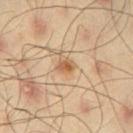Clinical impression: No biopsy was performed on this lesion — it was imaged during a full skin examination and was not determined to be concerning. Acquisition and patient details: Automated image analysis of the tile measured a lesion area of about 3 mm² and an outline eccentricity of about 0.65 (0 = round, 1 = elongated). The analysis additionally found roughly 11 lightness units darker than nearby skin and a lesion-to-skin contrast of about 7.5 (normalized; higher = more distinct). And it measured border irregularity of about 1.5 on a 0–10 scale. Located on the leg. The subject is a male approximately 45 years of age. This image is a 15 mm lesion crop taken from a total-body photograph. About 2 mm across. Imaged with cross-polarized lighting.